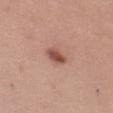A 15 mm close-up extracted from a 3D total-body photography capture. Located on the left thigh. The tile uses white-light illumination. The subject is a female in their mid- to late 20s.